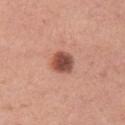Image and clinical context: This image is a 15 mm lesion crop taken from a total-body photograph. The recorded lesion diameter is about 3 mm. A female subject, about 30 years old. Imaged with white-light lighting. From the left thigh.